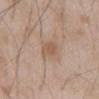{
  "image": {
    "source": "total-body photography crop",
    "field_of_view_mm": 15
  },
  "lesion_size": {
    "long_diameter_mm_approx": 3.0
  },
  "patient": {
    "sex": "male",
    "age_approx": 60
  },
  "lighting": "white-light",
  "site": "front of the torso",
  "automated_metrics": {
    "nevus_likeness_0_100": 15,
    "lesion_detection_confidence_0_100": 100
  }
}